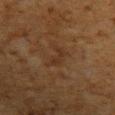The lesion was photographed on a routine skin check and not biopsied; there is no pathology result.
Captured under cross-polarized illumination.
A male patient aged 58–62.
From the left upper arm.
Cropped from a whole-body photographic skin survey; the tile spans about 15 mm.